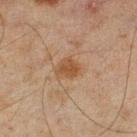Q: Was a biopsy performed?
A: total-body-photography surveillance lesion; no biopsy
Q: Lesion size?
A: ≈3 mm
Q: Automated lesion metrics?
A: two-axis asymmetry of about 0.2; a lesion-detection confidence of about 100/100
Q: What are the patient's age and sex?
A: male, aged around 45
Q: How was the tile lit?
A: cross-polarized illumination
Q: What kind of image is this?
A: 15 mm crop, total-body photography
Q: Where on the body is the lesion?
A: the left lower leg